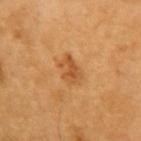Notes:
* biopsy status · imaged on a skin check; not biopsied
* anatomic site · the left upper arm
* acquisition · ~15 mm crop, total-body skin-cancer survey
* image-analysis metrics · an area of roughly 4.5 mm² and a symmetry-axis asymmetry near 0.4
* illumination · cross-polarized illumination
* patient · male, approximately 60 years of age
* lesion diameter · about 3 mm